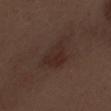| feature | finding |
|---|---|
| workup | catalogued during a skin exam; not biopsied |
| image source | ~15 mm tile from a whole-body skin photo |
| body site | the right forearm |
| subject | male, in their 70s |